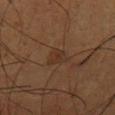lesion_size:
  long_diameter_mm_approx: 2.5
automated_metrics:
  vs_skin_darker_L: 5.0
  vs_skin_contrast_norm: 5.5
  border_irregularity_0_10: 3.5
  peripheral_color_asymmetry: 1.0
  nevus_likeness_0_100: 0
lighting: cross-polarized
patient:
  sex: male
  age_approx: 55
image:
  source: total-body photography crop
  field_of_view_mm: 15
site: front of the torso